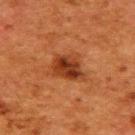Notes:
– workup: catalogued during a skin exam; not biopsied
– lighting: cross-polarized illumination
– image: total-body-photography crop, ~15 mm field of view
– location: the upper back
– image-analysis metrics: a lesion area of about 11 mm² and an outline eccentricity of about 0.65 (0 = round, 1 = elongated)
– size: about 4.5 mm
– patient: female, approximately 50 years of age